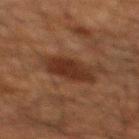Impression: The lesion was tiled from a total-body skin photograph and was not biopsied. Background: The total-body-photography lesion software estimated a within-lesion color-variation index near 2.5/10 and radial color variation of about 1. It also reported an automated nevus-likeness rating near 70 out of 100 and lesion-presence confidence of about 100/100. A roughly 15 mm field-of-view crop from a total-body skin photograph. A male subject, roughly 60 years of age. Imaged with cross-polarized lighting. Longest diameter approximately 4.5 mm. The lesion is on the mid back.